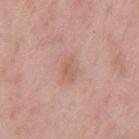Q: Is there a histopathology result?
A: imaged on a skin check; not biopsied
Q: What is the lesion's diameter?
A: ~3 mm (longest diameter)
Q: What lighting was used for the tile?
A: white-light
Q: How was this image acquired?
A: 15 mm crop, total-body photography
Q: Who is the patient?
A: male, about 55 years old
Q: What is the anatomic site?
A: the mid back
Q: What did automated image analysis measure?
A: a lesion area of about 4.5 mm², an eccentricity of roughly 0.8, and a shape-asymmetry score of about 0.3 (0 = symmetric); a normalized border contrast of about 5.5; a border-irregularity index near 3/10, a within-lesion color-variation index near 2/10, and peripheral color asymmetry of about 0.5